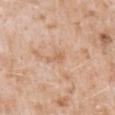Impression:
The lesion was tiled from a total-body skin photograph and was not biopsied.
Acquisition and patient details:
This is a white-light tile. The recorded lesion diameter is about 2.5 mm. The patient is a male in their 50s. The lesion is on the mid back. Cropped from a total-body skin-imaging series; the visible field is about 15 mm.